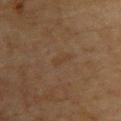Part of a total-body skin-imaging series; this lesion was reviewed on a skin check and was not flagged for biopsy.
A close-up tile cropped from a whole-body skin photograph, about 15 mm across.
The lesion is on the upper back.
Longest diameter approximately 2.5 mm.
This is a cross-polarized tile.
A female subject, approximately 80 years of age.